Located on the upper back; a 15 mm crop from a total-body photograph taken for skin-cancer surveillance; the patient is a male in their mid-70s:
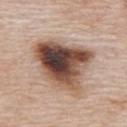{"automated_metrics": {"eccentricity": 0.55, "shape_asymmetry": 0.35, "cielab_L": 48, "cielab_a": 18, "cielab_b": 26, "vs_skin_contrast_norm": 14.0, "border_irregularity_0_10": 3.5, "color_variation_0_10": 10.0, "nevus_likeness_0_100": 5, "lesion_detection_confidence_0_100": 100}, "lighting": "white-light", "diagnosis": {"histopathology": "seborrheic keratosis", "malignancy": "benign", "taxonomic_path": ["Benign", "Benign epidermal proliferations", "Seborrheic keratosis"]}}